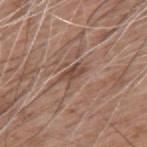biopsy_status: not biopsied; imaged during a skin examination
lesion_size:
  long_diameter_mm_approx: 3.5
image:
  source: total-body photography crop
  field_of_view_mm: 15
automated_metrics:
  area_mm2_approx: 4.5
  cielab_L: 47
  cielab_a: 19
  cielab_b: 26
  vs_skin_darker_L: 9.0
  vs_skin_contrast_norm: 6.5
  border_irregularity_0_10: 3.5
  color_variation_0_10: 3.0
  peripheral_color_asymmetry: 1.0
  nevus_likeness_0_100: 0
patient:
  sex: male
  age_approx: 60
lighting: white-light
site: upper back